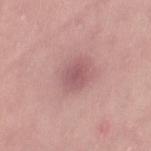image:
  source: total-body photography crop
  field_of_view_mm: 15
patient:
  sex: female
  age_approx: 25
automated_metrics:
  border_irregularity_0_10: 2.0
  color_variation_0_10: 2.5
  peripheral_color_asymmetry: 0.5
lighting: white-light
lesion_size:
  long_diameter_mm_approx: 3.0
site: left thigh